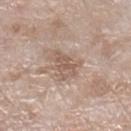biopsy_status: not biopsied; imaged during a skin examination
patient:
  sex: female
  age_approx: 75
lighting: white-light
lesion_size:
  long_diameter_mm_approx: 4.0
image:
  source: total-body photography crop
  field_of_view_mm: 15
automated_metrics:
  area_mm2_approx: 6.5
  eccentricity: 0.8
  vs_skin_darker_L: 9.0
  vs_skin_contrast_norm: 6.0
  color_variation_0_10: 3.0
  peripheral_color_asymmetry: 1.0
site: right lower leg